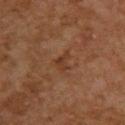Q: Was a biopsy performed?
A: imaged on a skin check; not biopsied
Q: Illumination type?
A: cross-polarized illumination
Q: What did automated image analysis measure?
A: a lesion area of about 3 mm², an eccentricity of roughly 0.75, and a symmetry-axis asymmetry near 0.5; a border-irregularity rating of about 5/10, a color-variation rating of about 1.5/10, and a peripheral color-asymmetry measure near 0.5; an automated nevus-likeness rating near 0 out of 100 and a detector confidence of about 100 out of 100 that the crop contains a lesion
Q: Lesion location?
A: the upper back
Q: Who is the patient?
A: female, aged approximately 60
Q: How large is the lesion?
A: ~2.5 mm (longest diameter)
Q: What kind of image is this?
A: total-body-photography crop, ~15 mm field of view